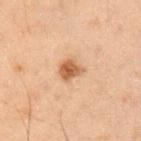Imaged during a routine full-body skin examination; the lesion was not biopsied and no histopathology is available.
A male subject, roughly 50 years of age.
Measured at roughly 2.5 mm in maximum diameter.
This is a cross-polarized tile.
A 15 mm close-up tile from a total-body photography series done for melanoma screening.
The lesion is located on the right upper arm.
An algorithmic analysis of the crop reported a lesion area of about 5 mm², an eccentricity of roughly 0.5, and a shape-asymmetry score of about 0.25 (0 = symmetric). The software also gave an average lesion color of about L≈44 a*≈18 b*≈30 (CIELAB), roughly 11 lightness units darker than nearby skin, and a normalized lesion–skin contrast near 9.